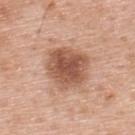Q: Is there a histopathology result?
A: imaged on a skin check; not biopsied
Q: What are the patient's age and sex?
A: male, aged 58–62
Q: Illumination type?
A: white-light illumination
Q: Lesion location?
A: the upper back
Q: What is the imaging modality?
A: ~15 mm crop, total-body skin-cancer survey